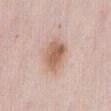Q: Is there a histopathology result?
A: imaged on a skin check; not biopsied
Q: Patient demographics?
A: female, approximately 60 years of age
Q: What lighting was used for the tile?
A: white-light illumination
Q: What did automated image analysis measure?
A: a shape eccentricity near 0.7 and two-axis asymmetry of about 0.2; a mean CIELAB color near L≈62 a*≈19 b*≈29; a color-variation rating of about 5/10 and peripheral color asymmetry of about 1.5; a classifier nevus-likeness of about 85/100 and a lesion-detection confidence of about 100/100
Q: How was this image acquired?
A: ~15 mm tile from a whole-body skin photo
Q: What is the anatomic site?
A: the front of the torso